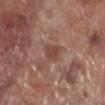Notes:
- notes — imaged on a skin check; not biopsied
- anatomic site — the right lower leg
- illumination — white-light illumination
- lesion size — ~2.5 mm (longest diameter)
- image — ~15 mm crop, total-body skin-cancer survey
- subject — male, about 70 years old